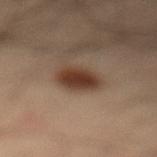Part of a total-body skin-imaging series; this lesion was reviewed on a skin check and was not flagged for biopsy. A male patient, aged 33 to 37. The total-body-photography lesion software estimated a shape eccentricity near 0.65. The analysis additionally found a lesion color around L≈34 a*≈17 b*≈24 in CIELAB and a normalized border contrast of about 11. From the left lower leg. A 15 mm close-up extracted from a 3D total-body photography capture.